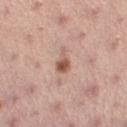Assessment:
Imaged during a routine full-body skin examination; the lesion was not biopsied and no histopathology is available.
Background:
A lesion tile, about 15 mm wide, cut from a 3D total-body photograph. On the left lower leg. The subject is a female roughly 25 years of age.